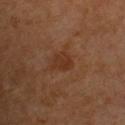Notes:
• follow-up — catalogued during a skin exam; not biopsied
• lesion diameter — ≈3 mm
• patient — female, approximately 70 years of age
• anatomic site — the upper back
• image — total-body-photography crop, ~15 mm field of view